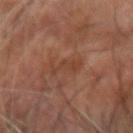Q: Was a biopsy performed?
A: catalogued during a skin exam; not biopsied